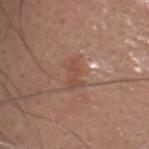The lesion was photographed on a routine skin check and not biopsied; there is no pathology result. On the head or neck. The patient is a male about 40 years old. An algorithmic analysis of the crop reported a footprint of about 4.5 mm², an outline eccentricity of about 0.9 (0 = round, 1 = elongated), and two-axis asymmetry of about 0.35. The software also gave a mean CIELAB color near L≈48 a*≈20 b*≈28, a lesion–skin lightness drop of about 7, and a lesion-to-skin contrast of about 5.5 (normalized; higher = more distinct). And it measured a nevus-likeness score of about 0/100. The lesion's longest dimension is about 3.5 mm. A close-up tile cropped from a whole-body skin photograph, about 15 mm across.